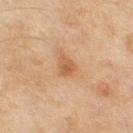Captured during whole-body skin photography for melanoma surveillance; the lesion was not biopsied.
The total-body-photography lesion software estimated an area of roughly 4 mm², a shape eccentricity near 0.7, and two-axis asymmetry of about 0.35. It also reported a classifier nevus-likeness of about 35/100.
Captured under cross-polarized illumination.
Located on the right thigh.
Cropped from a whole-body photographic skin survey; the tile spans about 15 mm.
The patient is a female aged around 60.
Approximately 3 mm at its widest.